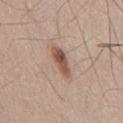Recorded during total-body skin imaging; not selected for excision or biopsy. Captured under white-light illumination. The lesion-visualizer software estimated a border-irregularity index near 3/10, a within-lesion color-variation index near 5/10, and radial color variation of about 1.5. The lesion is located on the front of the torso. The subject is a male about 45 years old. Longest diameter approximately 4 mm. A lesion tile, about 15 mm wide, cut from a 3D total-body photograph.